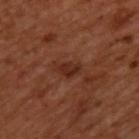The lesion was tiled from a total-body skin photograph and was not biopsied. Located on the upper back. Measured at roughly 2.5 mm in maximum diameter. Imaged with cross-polarized lighting. Cropped from a whole-body photographic skin survey; the tile spans about 15 mm. A male subject, in their 50s.